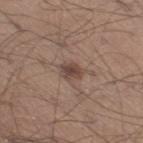Findings:
– follow-up: catalogued during a skin exam; not biopsied
– subject: male, aged around 30
– image: 15 mm crop, total-body photography
– lesion diameter: ~2.5 mm (longest diameter)
– location: the right thigh